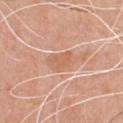This lesion was catalogued during total-body skin photography and was not selected for biopsy. This image is a 15 mm lesion crop taken from a total-body photograph. The lesion's longest dimension is about 4 mm. This is a white-light tile. The subject is a male aged 48 to 52. From the chest.